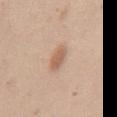No biopsy was performed on this lesion — it was imaged during a full skin examination and was not determined to be concerning. The lesion is located on the mid back. Captured under white-light illumination. A 15 mm close-up extracted from a 3D total-body photography capture. A female subject about 40 years old.